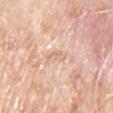Assessment:
The lesion was photographed on a routine skin check and not biopsied; there is no pathology result.
Context:
A male subject in their 80s. A lesion tile, about 15 mm wide, cut from a 3D total-body photograph. Captured under white-light illumination. Longest diameter approximately 3 mm. The lesion is located on the chest. An algorithmic analysis of the crop reported a shape eccentricity near 0.9. The software also gave a lesion-to-skin contrast of about 5 (normalized; higher = more distinct). And it measured internal color variation of about 0 on a 0–10 scale and a peripheral color-asymmetry measure near 0. It also reported a classifier nevus-likeness of about 0/100.